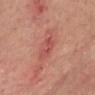The lesion was photographed on a routine skin check and not biopsied; there is no pathology result.
Cropped from a whole-body photographic skin survey; the tile spans about 15 mm.
The subject is a male in their mid-60s.
Located on the chest.
Imaged with white-light lighting.
The recorded lesion diameter is about 2.5 mm.